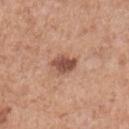{"biopsy_status": "not biopsied; imaged during a skin examination", "automated_metrics": {"area_mm2_approx": 5.0, "eccentricity": 0.6, "color_variation_0_10": 3.0, "peripheral_color_asymmetry": 1.0, "nevus_likeness_0_100": 80, "lesion_detection_confidence_0_100": 100}, "image": {"source": "total-body photography crop", "field_of_view_mm": 15}, "lighting": "white-light", "patient": {"sex": "male", "age_approx": 55}, "site": "chest"}